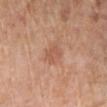The lesion was photographed on a routine skin check and not biopsied; there is no pathology result.
A female subject in their mid-50s.
The recorded lesion diameter is about 2.5 mm.
A lesion tile, about 15 mm wide, cut from a 3D total-body photograph.
Automated tile analysis of the lesion measured a footprint of about 4.5 mm², a shape eccentricity near 0.55, and two-axis asymmetry of about 0.15. The software also gave border irregularity of about 1.5 on a 0–10 scale and a peripheral color-asymmetry measure near 0.5. The analysis additionally found an automated nevus-likeness rating near 5 out of 100 and lesion-presence confidence of about 100/100.
The tile uses cross-polarized illumination.
Located on the left lower leg.